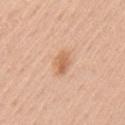{"biopsy_status": "not biopsied; imaged during a skin examination", "site": "left upper arm", "lesion_size": {"long_diameter_mm_approx": 3.0}, "image": {"source": "total-body photography crop", "field_of_view_mm": 15}, "patient": {"sex": "female", "age_approx": 40}, "lighting": "white-light"}